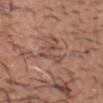Clinical impression: The lesion was photographed on a routine skin check and not biopsied; there is no pathology result. Clinical summary: On the head or neck. A 15 mm close-up extracted from a 3D total-body photography capture. The tile uses white-light illumination. Longest diameter approximately 4 mm. The patient is a male roughly 40 years of age. An algorithmic analysis of the crop reported a lesion area of about 8 mm², a shape eccentricity near 0.7, and a symmetry-axis asymmetry near 0.7. It also reported border irregularity of about 9.5 on a 0–10 scale, a color-variation rating of about 3.5/10, and peripheral color asymmetry of about 1.